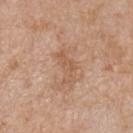No biopsy was performed on this lesion — it was imaged during a full skin examination and was not determined to be concerning.